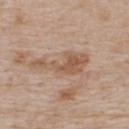Impression: This lesion was catalogued during total-body skin photography and was not selected for biopsy. Image and clinical context: This is a white-light tile. Located on the upper back. The total-body-photography lesion software estimated a footprint of about 12 mm², an eccentricity of roughly 0.95, and a shape-asymmetry score of about 0.6 (0 = symmetric). And it measured a mean CIELAB color near L≈56 a*≈18 b*≈30. And it measured lesion-presence confidence of about 100/100. A 15 mm close-up extracted from a 3D total-body photography capture. A male subject, aged approximately 75.